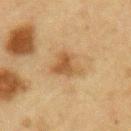Assessment:
Captured during whole-body skin photography for melanoma surveillance; the lesion was not biopsied.
Acquisition and patient details:
A close-up tile cropped from a whole-body skin photograph, about 15 mm across. The lesion is on the right upper arm. A male patient, aged 63–67.